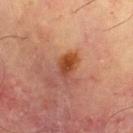{"biopsy_status": "not biopsied; imaged during a skin examination", "patient": {"sex": "male", "age_approx": 70}, "image": {"source": "total-body photography crop", "field_of_view_mm": 15}, "site": "chest", "lighting": "cross-polarized", "lesion_size": {"long_diameter_mm_approx": 3.0}}